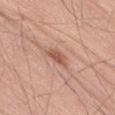Part of a total-body skin-imaging series; this lesion was reviewed on a skin check and was not flagged for biopsy.
A 15 mm crop from a total-body photograph taken for skin-cancer surveillance.
From the right thigh.
The recorded lesion diameter is about 2.5 mm.
A male patient, in their mid- to late 50s.